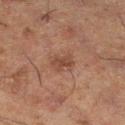<lesion>
  <biopsy_status>not biopsied; imaged during a skin examination</biopsy_status>
  <patient>
    <sex>male</sex>
    <age_approx>60</age_approx>
  </patient>
  <site>left leg</site>
  <image>
    <source>total-body photography crop</source>
    <field_of_view_mm>15</field_of_view_mm>
  </image>
</lesion>